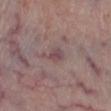lesion size — ≈3.5 mm | subject — male, approximately 65 years of age | anatomic site — the left lower leg | tile lighting — white-light illumination | TBP lesion metrics — an area of roughly 4 mm² and a symmetry-axis asymmetry near 0.45; border irregularity of about 5 on a 0–10 scale and peripheral color asymmetry of about 1 | image source — 15 mm crop, total-body photography.